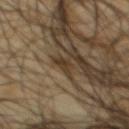Automated tile analysis of the lesion measured a nevus-likeness score of about 45/100 and lesion-presence confidence of about 55/100. A male patient, in their mid-60s. Captured under cross-polarized illumination. The recorded lesion diameter is about 3 mm. A 15 mm close-up extracted from a 3D total-body photography capture. On the back.